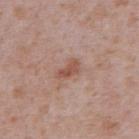follow-up = imaged on a skin check; not biopsied | acquisition = ~15 mm tile from a whole-body skin photo | site = the abdomen | lesion diameter = about 3 mm | patient = male, approximately 65 years of age.